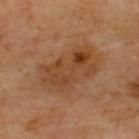Imaged during a routine full-body skin examination; the lesion was not biopsied and no histopathology is available.
The lesion-visualizer software estimated an automated nevus-likeness rating near 45 out of 100.
A close-up tile cropped from a whole-body skin photograph, about 15 mm across.
Measured at roughly 7 mm in maximum diameter.
On the upper back.
This is a cross-polarized tile.
A patient roughly 65 years of age.